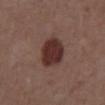Findings:
• workup · no biopsy performed (imaged during a skin exam)